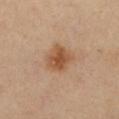{
  "biopsy_status": "not biopsied; imaged during a skin examination",
  "image": {
    "source": "total-body photography crop",
    "field_of_view_mm": 15
  },
  "site": "front of the torso",
  "patient": {
    "sex": "female",
    "age_approx": 40
  },
  "automated_metrics": {
    "cielab_L": 54,
    "cielab_a": 20,
    "cielab_b": 35,
    "vs_skin_darker_L": 10.0,
    "vs_skin_contrast_norm": 8.0,
    "peripheral_color_asymmetry": 1.0
  },
  "lighting": "cross-polarized",
  "lesion_size": {
    "long_diameter_mm_approx": 3.5
  }
}